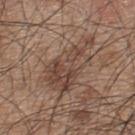About 7.5 mm across. Cropped from a total-body skin-imaging series; the visible field is about 15 mm. Imaged with white-light lighting. On the upper back. A male subject aged approximately 45.